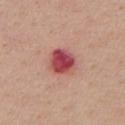illumination: white-light
subject: female, aged 38 to 42
lesion diameter: ≈3.5 mm
location: the front of the torso
imaging modality: ~15 mm crop, total-body skin-cancer survey